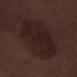image source = ~15 mm crop, total-body skin-cancer survey | TBP lesion metrics = a footprint of about 36 mm², a shape eccentricity near 0.7, and a symmetry-axis asymmetry near 0.15 | anatomic site = the right lower leg | lighting = white-light | subject = male, roughly 70 years of age.